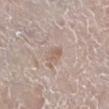{"automated_metrics": {"area_mm2_approx": 3.5, "eccentricity": 0.75, "color_variation_0_10": 2.5, "peripheral_color_asymmetry": 1.0}, "lesion_size": {"long_diameter_mm_approx": 2.5}, "lighting": "white-light", "patient": {"sex": "male", "age_approx": 80}, "site": "right lower leg", "image": {"source": "total-body photography crop", "field_of_view_mm": 15}}